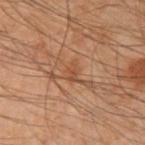Imaged with cross-polarized lighting. A 15 mm close-up extracted from a 3D total-body photography capture. A male subject, approximately 50 years of age. The recorded lesion diameter is about 2.5 mm. From the left forearm.